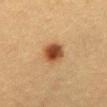biopsy_status: not biopsied; imaged during a skin examination
site: abdomen
image:
  source: total-body photography crop
  field_of_view_mm: 15
lesion_size:
  long_diameter_mm_approx: 3.0
lighting: cross-polarized
patient:
  sex: female
  age_approx: 40